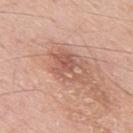follow-up: imaged on a skin check; not biopsied | size: ~4.5 mm (longest diameter) | tile lighting: white-light illumination | imaging modality: ~15 mm crop, total-body skin-cancer survey | image-analysis metrics: a footprint of about 8 mm², an eccentricity of roughly 0.75, and a shape-asymmetry score of about 0.4 (0 = symmetric); internal color variation of about 4.5 on a 0–10 scale and a peripheral color-asymmetry measure near 1.5 | subject: male, in their 50s | anatomic site: the mid back.